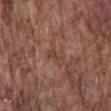Captured during whole-body skin photography for melanoma surveillance; the lesion was not biopsied. The lesion is on the back. The patient is a male about 75 years old. An algorithmic analysis of the crop reported lesion-presence confidence of about 90/100. The tile uses white-light illumination. Approximately 3 mm at its widest. A 15 mm crop from a total-body photograph taken for skin-cancer surveillance.